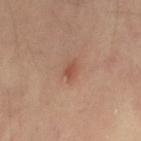{
  "biopsy_status": "not biopsied; imaged during a skin examination",
  "image": {
    "source": "total-body photography crop",
    "field_of_view_mm": 15
  },
  "site": "left thigh",
  "patient": {
    "sex": "female",
    "age_approx": 35
  },
  "lesion_size": {
    "long_diameter_mm_approx": 3.0
  }
}